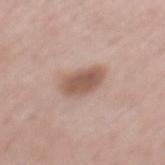Clinical impression:
No biopsy was performed on this lesion — it was imaged during a full skin examination and was not determined to be concerning.
Acquisition and patient details:
A male patient in their mid-50s. A region of skin cropped from a whole-body photographic capture, roughly 15 mm wide. On the back.